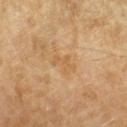Assessment: Recorded during total-body skin imaging; not selected for excision or biopsy. Acquisition and patient details: A lesion tile, about 15 mm wide, cut from a 3D total-body photograph. Imaged with cross-polarized lighting. From the arm. A female subject approximately 70 years of age. An algorithmic analysis of the crop reported a lesion area of about 4.5 mm² and an outline eccentricity of about 0.85 (0 = round, 1 = elongated). The analysis additionally found a mean CIELAB color near L≈61 a*≈19 b*≈42 and about 6 CIELAB-L* units darker than the surrounding skin. The software also gave a border-irregularity index near 6/10, a within-lesion color-variation index near 1/10, and a peripheral color-asymmetry measure near 0. The software also gave an automated nevus-likeness rating near 0 out of 100 and a detector confidence of about 100 out of 100 that the crop contains a lesion.